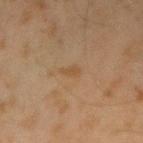Assessment: No biopsy was performed on this lesion — it was imaged during a full skin examination and was not determined to be concerning. Acquisition and patient details: Located on the left forearm. A region of skin cropped from a whole-body photographic capture, roughly 15 mm wide. A male subject, about 45 years old. Imaged with cross-polarized lighting. About 2.5 mm across.